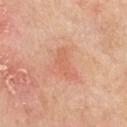Clinical summary: The recorded lesion diameter is about 4 mm. Imaged with white-light lighting. The subject is a female aged 68 to 72. On the chest. A close-up tile cropped from a whole-body skin photograph, about 15 mm across.